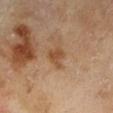The lesion was photographed on a routine skin check and not biopsied; there is no pathology result.
On the leg.
An algorithmic analysis of the crop reported a lesion color around L≈50 a*≈21 b*≈34 in CIELAB, about 9 CIELAB-L* units darker than the surrounding skin, and a normalized lesion–skin contrast near 7. It also reported border irregularity of about 4 on a 0–10 scale, a color-variation rating of about 1.5/10, and a peripheral color-asymmetry measure near 0.5. The software also gave an automated nevus-likeness rating near 5 out of 100 and lesion-presence confidence of about 100/100.
The subject is a male roughly 65 years of age.
A 15 mm crop from a total-body photograph taken for skin-cancer surveillance.
Measured at roughly 3 mm in maximum diameter.